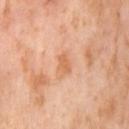No biopsy was performed on this lesion — it was imaged during a full skin examination and was not determined to be concerning.
The recorded lesion diameter is about 2.5 mm.
The lesion is located on the leg.
A female patient about 55 years old.
An algorithmic analysis of the crop reported a lesion area of about 3 mm² and two-axis asymmetry of about 0.25. It also reported a lesion color around L≈65 a*≈25 b*≈39 in CIELAB and roughly 8 lightness units darker than nearby skin. The analysis additionally found a border-irregularity rating of about 2.5/10, a color-variation rating of about 0.5/10, and radial color variation of about 0.
A 15 mm crop from a total-body photograph taken for skin-cancer surveillance.